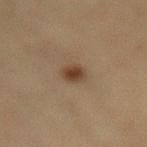Findings:
• follow-up — total-body-photography surveillance lesion; no biopsy
• anatomic site — the lower back
• lesion size — ~2.5 mm (longest diameter)
• lighting — cross-polarized illumination
• subject — female, aged 38 to 42
• acquisition — ~15 mm crop, total-body skin-cancer survey